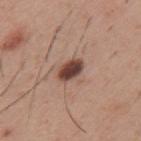Impression: Imaged during a routine full-body skin examination; the lesion was not biopsied and no histopathology is available. Context: The lesion is located on the back. A roughly 15 mm field-of-view crop from a total-body skin photograph. Automated tile analysis of the lesion measured a lesion area of about 5 mm² and a shape eccentricity near 0.8. The software also gave a border-irregularity index near 1.5/10, a color-variation rating of about 4/10, and radial color variation of about 1.5. It also reported lesion-presence confidence of about 100/100. Imaged with white-light lighting. The subject is a male aged 28 to 32.